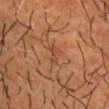<lesion>
<biopsy_status>not biopsied; imaged during a skin examination</biopsy_status>
<patient>
  <sex>male</sex>
  <age_approx>40</age_approx>
</patient>
<lesion_size>
  <long_diameter_mm_approx>2.5</long_diameter_mm_approx>
</lesion_size>
<automated_metrics>
  <area_mm2_approx>2.5</area_mm2_approx>
  <eccentricity>0.8</eccentricity>
  <shape_asymmetry>0.55</shape_asymmetry>
  <border_irregularity_0_10>6.0</border_irregularity_0_10>
  <color_variation_0_10>0.0</color_variation_0_10>
  <peripheral_color_asymmetry>0.0</peripheral_color_asymmetry>
  <nevus_likeness_0_100>0</nevus_likeness_0_100>
  <lesion_detection_confidence_0_100>70</lesion_detection_confidence_0_100>
</automated_metrics>
<lighting>cross-polarized</lighting>
<site>head or neck</site>
<image>
  <source>total-body photography crop</source>
  <field_of_view_mm>15</field_of_view_mm>
</image>
</lesion>